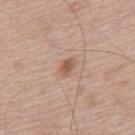From the upper back. This image is a 15 mm lesion crop taken from a total-body photograph. Automated image analysis of the tile measured a lesion area of about 3.5 mm², an eccentricity of roughly 0.8, and a symmetry-axis asymmetry near 0.2. And it measured internal color variation of about 3 on a 0–10 scale. The patient is a male aged around 80. Imaged with white-light lighting. Measured at roughly 2.5 mm in maximum diameter.